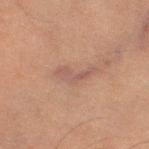notes: total-body-photography surveillance lesion; no biopsy
acquisition: 15 mm crop, total-body photography
diameter: about 4.5 mm
tile lighting: cross-polarized
body site: the leg
patient: female, approximately 60 years of age